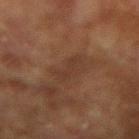- workup · no biopsy performed (imaged during a skin exam)
- patient · male, roughly 75 years of age
- image source · ~15 mm tile from a whole-body skin photo
- location · the right upper arm
- diameter · ≈4 mm